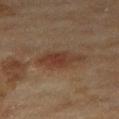| feature | finding |
|---|---|
| follow-up | no biopsy performed (imaged during a skin exam) |
| diameter | about 5.5 mm |
| illumination | cross-polarized illumination |
| acquisition | total-body-photography crop, ~15 mm field of view |
| automated lesion analysis | a lesion area of about 10 mm², an eccentricity of roughly 0.9, and a shape-asymmetry score of about 0.25 (0 = symmetric); an average lesion color of about L≈34 a*≈18 b*≈26 (CIELAB) and a lesion–skin lightness drop of about 8; an automated nevus-likeness rating near 90 out of 100 and lesion-presence confidence of about 100/100 |
| subject | female, aged approximately 60 |
| body site | the right thigh |